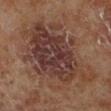Impression: Captured during whole-body skin photography for melanoma surveillance; the lesion was not biopsied. Clinical summary: The subject is a male in their 70s. The lesion is on the left lower leg. A 15 mm close-up tile from a total-body photography series done for melanoma screening.